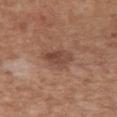biopsy_status: not biopsied; imaged during a skin examination
site: chest
lesion_size:
  long_diameter_mm_approx: 3.5
patient:
  sex: female
  age_approx: 35
image:
  source: total-body photography crop
  field_of_view_mm: 15